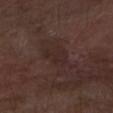Impression: The lesion was tiled from a total-body skin photograph and was not biopsied. Acquisition and patient details: A male patient in their mid-70s. A 15 mm close-up tile from a total-body photography series done for melanoma screening. The tile uses white-light illumination. Located on the left forearm. The recorded lesion diameter is about 3 mm.